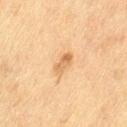Clinical impression:
The lesion was tiled from a total-body skin photograph and was not biopsied.
Background:
A female patient, aged approximately 55. On the leg. Automated tile analysis of the lesion measured a detector confidence of about 100 out of 100 that the crop contains a lesion. Imaged with cross-polarized lighting. A close-up tile cropped from a whole-body skin photograph, about 15 mm across.